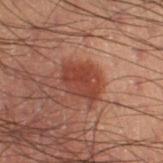notes = no biopsy performed (imaged during a skin exam); acquisition = ~15 mm crop, total-body skin-cancer survey; subject = male, about 55 years old; location = the leg.